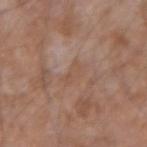biopsy status: no biopsy performed (imaged during a skin exam) | diameter: ~3.5 mm (longest diameter) | subject: male, aged approximately 55 | automated lesion analysis: a lesion color around L≈52 a*≈18 b*≈29 in CIELAB; a border-irregularity index near 4/10 and a peripheral color-asymmetry measure near 0.5 | image: total-body-photography crop, ~15 mm field of view | illumination: white-light illumination | location: the arm.